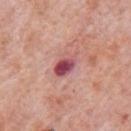Impression:
The lesion was tiled from a total-body skin photograph and was not biopsied.
Acquisition and patient details:
On the chest. A male subject, in their 80s. Imaged with white-light lighting. About 3.5 mm across. Cropped from a whole-body photographic skin survey; the tile spans about 15 mm.